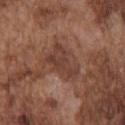biopsy status = no biopsy performed (imaged during a skin exam) | lighting = white-light | image source = ~15 mm crop, total-body skin-cancer survey | patient = male, in their mid- to late 70s | body site = the chest | diameter = about 4.5 mm.